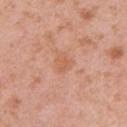Imaged during a routine full-body skin examination; the lesion was not biopsied and no histopathology is available.
The lesion is on the arm.
Imaged with white-light lighting.
Cropped from a whole-body photographic skin survey; the tile spans about 15 mm.
Measured at roughly 2.5 mm in maximum diameter.
A female subject, in their 40s.